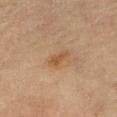notes — total-body-photography surveillance lesion; no biopsy
body site — the right lower leg
subject — female, aged approximately 80
tile lighting — cross-polarized illumination
automated lesion analysis — a footprint of about 3.5 mm², a shape eccentricity near 0.85, and a symmetry-axis asymmetry near 0.25; about 6 CIELAB-L* units darker than the surrounding skin and a lesion-to-skin contrast of about 6 (normalized; higher = more distinct); a border-irregularity rating of about 2.5/10, a within-lesion color-variation index near 2/10, and peripheral color asymmetry of about 0.5
image source — total-body-photography crop, ~15 mm field of view